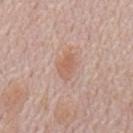| field | value |
|---|---|
| workup | imaged on a skin check; not biopsied |
| size | ≈3.5 mm |
| imaging modality | total-body-photography crop, ~15 mm field of view |
| patient | male, roughly 70 years of age |
| body site | the mid back |
| illumination | white-light illumination |